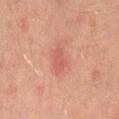Impression: Imaged during a routine full-body skin examination; the lesion was not biopsied and no histopathology is available. Acquisition and patient details: This image is a 15 mm lesion crop taken from a total-body photograph. Imaged with cross-polarized lighting. The lesion is located on the back. The patient is a male about 45 years old.